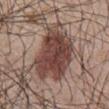Recorded during total-body skin imaging; not selected for excision or biopsy. The lesion-visualizer software estimated an area of roughly 31 mm², a shape eccentricity near 0.9, and two-axis asymmetry of about 0.25. The analysis additionally found a border-irregularity rating of about 3.5/10, internal color variation of about 5 on a 0–10 scale, and peripheral color asymmetry of about 1.5. The tile uses white-light illumination. The patient is a male roughly 45 years of age. Longest diameter approximately 9.5 mm. A close-up tile cropped from a whole-body skin photograph, about 15 mm across. On the mid back.